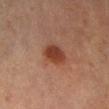follow-up: total-body-photography surveillance lesion; no biopsy
acquisition: ~15 mm tile from a whole-body skin photo
location: the right lower leg
subject: male, aged 68 to 72
lighting: cross-polarized illumination